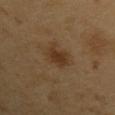The lesion was tiled from a total-body skin photograph and was not biopsied. On the arm. Measured at roughly 3 mm in maximum diameter. A male patient, aged 58–62. This is a cross-polarized tile. A 15 mm crop from a total-body photograph taken for skin-cancer surveillance. Automated tile analysis of the lesion measured a lesion area of about 6 mm², a shape eccentricity near 0.45, and two-axis asymmetry of about 0.2.